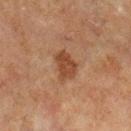biopsy_status: not biopsied; imaged during a skin examination
patient:
  sex: male
  age_approx: 65
lighting: cross-polarized
site: left lower leg
image:
  source: total-body photography crop
  field_of_view_mm: 15
lesion_size:
  long_diameter_mm_approx: 3.5